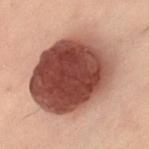biopsy status — no biopsy performed (imaged during a skin exam)
imaging modality — 15 mm crop, total-body photography
subject — female, aged 58–62
anatomic site — the right leg